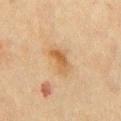<lesion>
<biopsy_status>not biopsied; imaged during a skin examination</biopsy_status>
<site>chest</site>
<patient>
  <sex>male</sex>
  <age_approx>70</age_approx>
</patient>
<lesion_size>
  <long_diameter_mm_approx>3.5</long_diameter_mm_approx>
</lesion_size>
<image>
  <source>total-body photography crop</source>
  <field_of_view_mm>15</field_of_view_mm>
</image>
<lighting>cross-polarized</lighting>
</lesion>